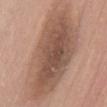* biopsy status — catalogued during a skin exam; not biopsied
* site — the abdomen
* image-analysis metrics — an outline eccentricity of about 0.9 (0 = round, 1 = elongated) and a symmetry-axis asymmetry near 0.2; an average lesion color of about L≈52 a*≈19 b*≈27 (CIELAB) and a lesion-to-skin contrast of about 8.5 (normalized; higher = more distinct)
* subject — male, aged approximately 60
* acquisition — 15 mm crop, total-body photography